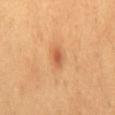Imaged during a routine full-body skin examination; the lesion was not biopsied and no histopathology is available.
From the mid back.
The tile uses cross-polarized illumination.
The lesion's longest dimension is about 2.5 mm.
A 15 mm close-up extracted from a 3D total-body photography capture.
The patient is a female approximately 40 years of age.
Automated tile analysis of the lesion measured a lesion area of about 3 mm², an outline eccentricity of about 0.85 (0 = round, 1 = elongated), and a symmetry-axis asymmetry near 0.25. And it measured a lesion color around L≈58 a*≈27 b*≈40 in CIELAB and a normalized border contrast of about 7. The software also gave a border-irregularity rating of about 2/10, internal color variation of about 3 on a 0–10 scale, and radial color variation of about 1.